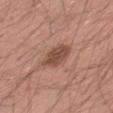{
  "biopsy_status": "not biopsied; imaged during a skin examination",
  "patient": {
    "sex": "male",
    "age_approx": 40
  },
  "image": {
    "source": "total-body photography crop",
    "field_of_view_mm": 15
  },
  "site": "right thigh"
}